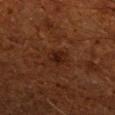notes = catalogued during a skin exam; not biopsied
imaging modality = total-body-photography crop, ~15 mm field of view
patient = male, aged around 60
diameter = ≈2.5 mm
body site = the right upper arm
lighting = cross-polarized illumination